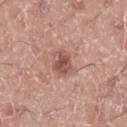Part of a total-body skin-imaging series; this lesion was reviewed on a skin check and was not flagged for biopsy.
Imaged with white-light lighting.
The patient is a male aged approximately 20.
The lesion's longest dimension is about 2.5 mm.
The lesion is located on the leg.
Cropped from a total-body skin-imaging series; the visible field is about 15 mm.